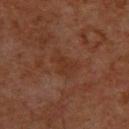Captured during whole-body skin photography for melanoma surveillance; the lesion was not biopsied. The lesion-visualizer software estimated a lesion area of about 7 mm², an outline eccentricity of about 0.7 (0 = round, 1 = elongated), and two-axis asymmetry of about 0.3. The analysis additionally found a lesion color around L≈29 a*≈20 b*≈26 in CIELAB, about 4 CIELAB-L* units darker than the surrounding skin, and a normalized lesion–skin contrast near 5. And it measured a lesion-detection confidence of about 100/100. The recorded lesion diameter is about 3.5 mm. The tile uses cross-polarized illumination. From the chest. Cropped from a total-body skin-imaging series; the visible field is about 15 mm. The patient is a male roughly 60 years of age.